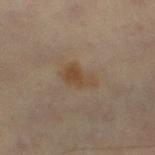Findings:
• acquisition — 15 mm crop, total-body photography
• site — the left thigh
• illumination — cross-polarized
• diameter — ≈3.5 mm
• subject — male, about 60 years old